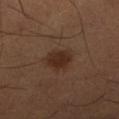biopsy status: catalogued during a skin exam; not biopsied
image source: ~15 mm crop, total-body skin-cancer survey
lesion diameter: ≈3 mm
tile lighting: cross-polarized
image-analysis metrics: an area of roughly 6.5 mm², an eccentricity of roughly 0.65, and a shape-asymmetry score of about 0.2 (0 = symmetric); an average lesion color of about L≈27 a*≈17 b*≈25 (CIELAB), roughly 8 lightness units darker than nearby skin, and a normalized border contrast of about 9
anatomic site: the leg
subject: male, aged around 50